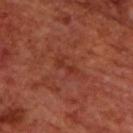The lesion was photographed on a routine skin check and not biopsied; there is no pathology result. A roughly 15 mm field-of-view crop from a total-body skin photograph. Approximately 3 mm at its widest. Located on the upper back. The lesion-visualizer software estimated a border-irregularity rating of about 4.5/10, internal color variation of about 0 on a 0–10 scale, and a peripheral color-asymmetry measure near 0. And it measured a nevus-likeness score of about 0/100 and a detector confidence of about 100 out of 100 that the crop contains a lesion. A male patient about 70 years old.